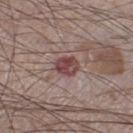Clinical impression: No biopsy was performed on this lesion — it was imaged during a full skin examination and was not determined to be concerning. Clinical summary: Cropped from a total-body skin-imaging series; the visible field is about 15 mm. From the right lower leg. A male patient about 75 years old. This is a white-light tile. Approximately 3 mm at its widest. Automated tile analysis of the lesion measured a lesion color around L≈44 a*≈21 b*≈18 in CIELAB, roughly 12 lightness units darker than nearby skin, and a normalized lesion–skin contrast near 9. It also reported a classifier nevus-likeness of about 10/100 and a detector confidence of about 100 out of 100 that the crop contains a lesion.